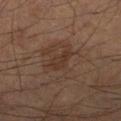<case>
<biopsy_status>not biopsied; imaged during a skin examination</biopsy_status>
<automated_metrics>
  <area_mm2_approx>4.5</area_mm2_approx>
  <shape_asymmetry>0.35</shape_asymmetry>
</automated_metrics>
<lesion_size>
  <long_diameter_mm_approx>3.0</long_diameter_mm_approx>
</lesion_size>
<image>
  <source>total-body photography crop</source>
  <field_of_view_mm>15</field_of_view_mm>
</image>
<site>right lower leg</site>
<lighting>cross-polarized</lighting>
<patient>
  <sex>male</sex>
  <age_approx>55</age_approx>
</patient>
</case>